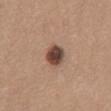follow-up — total-body-photography surveillance lesion; no biopsy | site — the abdomen | lesion diameter — ≈3 mm | patient — female, approximately 35 years of age | image source — ~15 mm crop, total-body skin-cancer survey | lighting — white-light.